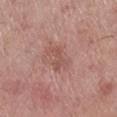No biopsy was performed on this lesion — it was imaged during a full skin examination and was not determined to be concerning. The patient is a female in their 70s. Located on the right lower leg. The lesion's longest dimension is about 3 mm. Automated tile analysis of the lesion measured a lesion area of about 3.5 mm², an eccentricity of roughly 0.85, and two-axis asymmetry of about 0.5. The software also gave a border-irregularity rating of about 6/10, a color-variation rating of about 0.5/10, and peripheral color asymmetry of about 0. Imaged with white-light lighting. A 15 mm close-up extracted from a 3D total-body photography capture.